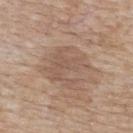biopsy status = total-body-photography surveillance lesion; no biopsy
body site = the upper back
patient = female, aged approximately 75
imaging modality = total-body-photography crop, ~15 mm field of view